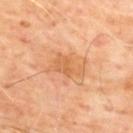Part of a total-body skin-imaging series; this lesion was reviewed on a skin check and was not flagged for biopsy. This is a cross-polarized tile. A male patient aged 63 to 67. The lesion is located on the upper back. A region of skin cropped from a whole-body photographic capture, roughly 15 mm wide. Automated image analysis of the tile measured an area of roughly 7.5 mm², a shape eccentricity near 0.85, and a shape-asymmetry score of about 0.45 (0 = symmetric). And it measured a detector confidence of about 100 out of 100 that the crop contains a lesion.